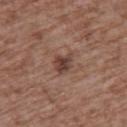<record>
  <biopsy_status>not biopsied; imaged during a skin examination</biopsy_status>
  <lighting>white-light</lighting>
  <patient>
    <sex>female</sex>
    <age_approx>75</age_approx>
  </patient>
  <lesion_size>
    <long_diameter_mm_approx>2.5</long_diameter_mm_approx>
  </lesion_size>
  <image>
    <source>total-body photography crop</source>
    <field_of_view_mm>15</field_of_view_mm>
  </image>
  <automated_metrics>
    <area_mm2_approx>4.5</area_mm2_approx>
    <eccentricity>0.6</eccentricity>
    <shape_asymmetry>0.3</shape_asymmetry>
    <cielab_L>41</cielab_L>
    <cielab_a>19</cielab_a>
    <cielab_b>23</cielab_b>
    <vs_skin_darker_L>11.0</vs_skin_darker_L>
    <vs_skin_contrast_norm>9.0</vs_skin_contrast_norm>
  </automated_metrics>
  <site>back</site>
</record>